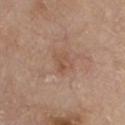patient: male, approximately 80 years of age
image-analysis metrics: an outline eccentricity of about 0.65 (0 = round, 1 = elongated) and two-axis asymmetry of about 0.3; about 6 CIELAB-L* units darker than the surrounding skin and a normalized border contrast of about 5
tile lighting: white-light
lesion diameter: ≈2.5 mm
image source: 15 mm crop, total-body photography
location: the chest